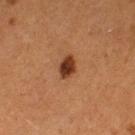This lesion was catalogued during total-body skin photography and was not selected for biopsy.
The subject is a female about 50 years old.
Located on the left thigh.
Imaged with cross-polarized lighting.
A 15 mm close-up tile from a total-body photography series done for melanoma screening.
Automated tile analysis of the lesion measured a border-irregularity rating of about 2/10 and a peripheral color-asymmetry measure near 1.5.